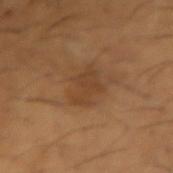workup: no biopsy performed (imaged during a skin exam)
lighting: cross-polarized illumination
automated metrics: an area of roughly 8.5 mm² and a shape-asymmetry score of about 0.35 (0 = symmetric)
acquisition: ~15 mm crop, total-body skin-cancer survey
subject: male, in their mid-60s
anatomic site: the right forearm
diameter: about 4 mm